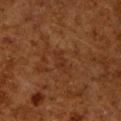Imaged during a routine full-body skin examination; the lesion was not biopsied and no histopathology is available.
The total-body-photography lesion software estimated an area of roughly 3 mm², an eccentricity of roughly 0.85, and a shape-asymmetry score of about 0.45 (0 = symmetric). The software also gave an average lesion color of about L≈25 a*≈19 b*≈27 (CIELAB) and a lesion-to-skin contrast of about 4.5 (normalized; higher = more distinct). The software also gave a classifier nevus-likeness of about 0/100.
Located on the right upper arm.
Longest diameter approximately 2.5 mm.
A 15 mm close-up extracted from a 3D total-body photography capture.
This is a cross-polarized tile.
A male subject, aged 58–62.